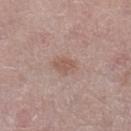Case summary:
– follow-up: imaged on a skin check; not biopsied
– imaging modality: total-body-photography crop, ~15 mm field of view
– patient: female, aged approximately 65
– diameter: about 3 mm
– location: the leg
– automated lesion analysis: a footprint of about 4 mm², an eccentricity of roughly 0.7, and two-axis asymmetry of about 0.25; a lesion color around L≈55 a*≈18 b*≈25 in CIELAB, about 7 CIELAB-L* units darker than the surrounding skin, and a normalized lesion–skin contrast near 6; a within-lesion color-variation index near 1.5/10; a nevus-likeness score of about 15/100 and a detector confidence of about 100 out of 100 that the crop contains a lesion
– tile lighting: white-light